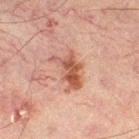Part of a total-body skin-imaging series; this lesion was reviewed on a skin check and was not flagged for biopsy.
The lesion is located on the leg.
The lesion-visualizer software estimated a border-irregularity index near 5.5/10 and radial color variation of about 1.
A 15 mm crop from a total-body photograph taken for skin-cancer surveillance.
The tile uses cross-polarized illumination.
A male patient, aged approximately 60.